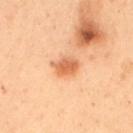Impression:
Captured during whole-body skin photography for melanoma surveillance; the lesion was not biopsied.
Clinical summary:
A region of skin cropped from a whole-body photographic capture, roughly 15 mm wide. The lesion is located on the mid back. The lesion-visualizer software estimated a lesion area of about 6 mm², an outline eccentricity of about 0.6 (0 = round, 1 = elongated), and two-axis asymmetry of about 0.2. The analysis additionally found an average lesion color of about L≈56 a*≈24 b*≈36 (CIELAB), about 11 CIELAB-L* units darker than the surrounding skin, and a normalized border contrast of about 7.5. And it measured a nevus-likeness score of about 100/100 and lesion-presence confidence of about 100/100. A female subject aged 48 to 52.